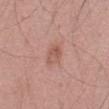From the abdomen.
The subject is a male in their mid- to late 50s.
Cropped from a whole-body photographic skin survey; the tile spans about 15 mm.